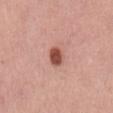Impression: The lesion was tiled from a total-body skin photograph and was not biopsied. Image and clinical context: The lesion is on the mid back. Automated tile analysis of the lesion measured a lesion color around L≈51 a*≈27 b*≈28 in CIELAB and a lesion–skin lightness drop of about 16. And it measured border irregularity of about 1.5 on a 0–10 scale, internal color variation of about 3 on a 0–10 scale, and peripheral color asymmetry of about 1. It also reported a classifier nevus-likeness of about 100/100. This is a white-light tile. The subject is a male aged 53 to 57. Cropped from a whole-body photographic skin survey; the tile spans about 15 mm.